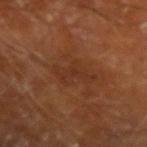notes=catalogued during a skin exam; not biopsied | patient=male, roughly 65 years of age | location=the arm | acquisition=~15 mm tile from a whole-body skin photo.